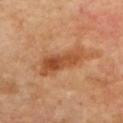Q: Is there a histopathology result?
A: imaged on a skin check; not biopsied
Q: Automated lesion metrics?
A: a footprint of about 13 mm² and an outline eccentricity of about 0.9 (0 = round, 1 = elongated); a nevus-likeness score of about 35/100 and lesion-presence confidence of about 100/100
Q: What kind of image is this?
A: total-body-photography crop, ~15 mm field of view
Q: Patient demographics?
A: male, aged 63 to 67
Q: Illumination type?
A: cross-polarized
Q: What is the lesion's diameter?
A: ~6 mm (longest diameter)